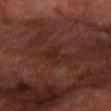  biopsy_status: not biopsied; imaged during a skin examination
  lesion_size:
    long_diameter_mm_approx: 3.0
  site: left forearm
  patient:
    age_approx: 65
  image:
    source: total-body photography crop
    field_of_view_mm: 15